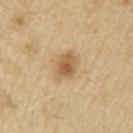  biopsy_status: not biopsied; imaged during a skin examination
  lesion_size:
    long_diameter_mm_approx: 4.0
  site: right upper arm
  image:
    source: total-body photography crop
    field_of_view_mm: 15
  patient:
    sex: male
    age_approx: 70
  lighting: white-light